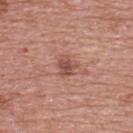Recorded during total-body skin imaging; not selected for excision or biopsy. An algorithmic analysis of the crop reported border irregularity of about 3.5 on a 0–10 scale and peripheral color asymmetry of about 1. From the upper back. Longest diameter approximately 2.5 mm. A male patient, in their 70s. A lesion tile, about 15 mm wide, cut from a 3D total-body photograph. The tile uses white-light illumination.